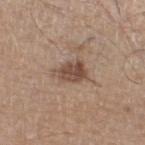Assessment:
The lesion was photographed on a routine skin check and not biopsied; there is no pathology result.